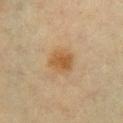Impression: Recorded during total-body skin imaging; not selected for excision or biopsy. Clinical summary: The patient is a female in their mid- to late 40s. This is a cross-polarized tile. The lesion is located on the chest. Longest diameter approximately 3 mm. An algorithmic analysis of the crop reported a footprint of about 7 mm², an outline eccentricity of about 0.4 (0 = round, 1 = elongated), and a shape-asymmetry score of about 0.2 (0 = symmetric). The analysis additionally found an automated nevus-likeness rating near 95 out of 100 and lesion-presence confidence of about 100/100. A region of skin cropped from a whole-body photographic capture, roughly 15 mm wide.